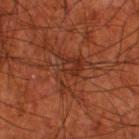Q: Was a biopsy performed?
A: total-body-photography surveillance lesion; no biopsy
Q: How was this image acquired?
A: ~15 mm crop, total-body skin-cancer survey
Q: What is the lesion's diameter?
A: ~4.5 mm (longest diameter)
Q: What are the patient's age and sex?
A: male, approximately 70 years of age
Q: What lighting was used for the tile?
A: cross-polarized illumination
Q: Lesion location?
A: the right thigh
Q: What did automated image analysis measure?
A: a footprint of about 8.5 mm², an eccentricity of roughly 0.7, and a symmetry-axis asymmetry near 0.55; a lesion-detection confidence of about 90/100